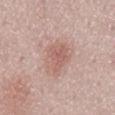follow-up = imaged on a skin check; not biopsied | diameter = ≈5 mm | lighting = white-light | acquisition = ~15 mm tile from a whole-body skin photo | site = the mid back | patient = female, approximately 50 years of age.